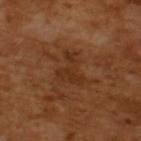biopsy status = imaged on a skin check; not biopsied
acquisition = ~15 mm tile from a whole-body skin photo
automated lesion analysis = a lesion color around L≈31 a*≈21 b*≈31 in CIELAB, a lesion–skin lightness drop of about 6, and a normalized lesion–skin contrast near 6; a border-irregularity rating of about 7/10, a within-lesion color-variation index near 1.5/10, and a peripheral color-asymmetry measure near 0.5
lesion size = about 4.5 mm
patient = male, in their mid- to late 60s